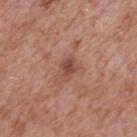{"biopsy_status": "not biopsied; imaged during a skin examination", "lesion_size": {"long_diameter_mm_approx": 2.5}, "site": "back", "image": {"source": "total-body photography crop", "field_of_view_mm": 15}, "patient": {"sex": "male", "age_approx": 65}, "lighting": "white-light"}